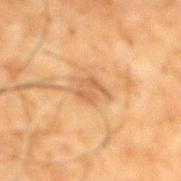workup: catalogued during a skin exam; not biopsied | automated lesion analysis: an area of roughly 4.5 mm², an eccentricity of roughly 0.65, and a shape-asymmetry score of about 0.4 (0 = symmetric); a mean CIELAB color near L≈49 a*≈19 b*≈33, roughly 8 lightness units darker than nearby skin, and a lesion-to-skin contrast of about 6 (normalized; higher = more distinct); border irregularity of about 4.5 on a 0–10 scale, internal color variation of about 2.5 on a 0–10 scale, and a peripheral color-asymmetry measure near 1; a classifier nevus-likeness of about 0/100 and lesion-presence confidence of about 100/100 | diameter: about 3 mm | imaging modality: ~15 mm tile from a whole-body skin photo | patient: male, in their mid- to late 60s | illumination: cross-polarized illumination | body site: the mid back.